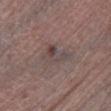biopsy status: imaged on a skin check; not biopsied | automated metrics: a lesion area of about 6.5 mm², a shape eccentricity near 0.85, and two-axis asymmetry of about 0.5 | patient: male, approximately 70 years of age | imaging modality: ~15 mm tile from a whole-body skin photo | lighting: white-light | lesion size: ≈4 mm | site: the left lower leg.